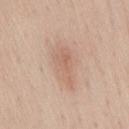This lesion was catalogued during total-body skin photography and was not selected for biopsy. The total-body-photography lesion software estimated an average lesion color of about L≈63 a*≈19 b*≈29 (CIELAB), a lesion–skin lightness drop of about 7, and a normalized lesion–skin contrast near 5. And it measured a nevus-likeness score of about 30/100 and lesion-presence confidence of about 100/100. Longest diameter approximately 5 mm. The lesion is on the lower back. The patient is a male aged approximately 75. The tile uses white-light illumination. Cropped from a total-body skin-imaging series; the visible field is about 15 mm.